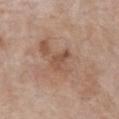Recorded during total-body skin imaging; not selected for excision or biopsy.
The tile uses white-light illumination.
Cropped from a whole-body photographic skin survey; the tile spans about 15 mm.
A female patient, approximately 85 years of age or older.
Longest diameter approximately 3 mm.
On the chest.